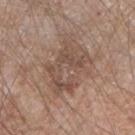workup: imaged on a skin check; not biopsied | image-analysis metrics: an eccentricity of roughly 0.75 and a symmetry-axis asymmetry near 0.4; a border-irregularity rating of about 8/10, a color-variation rating of about 3.5/10, and a peripheral color-asymmetry measure near 1; a nevus-likeness score of about 0/100 | image: ~15 mm tile from a whole-body skin photo | illumination: white-light illumination | lesion diameter: ≈6 mm | location: the right forearm | patient: male, about 75 years old.